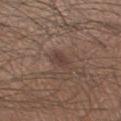• biopsy status: no biopsy performed (imaged during a skin exam)
• subject: male, in their mid- to late 40s
• location: the leg
• lesion size: ~2.5 mm (longest diameter)
• acquisition: ~15 mm tile from a whole-body skin photo